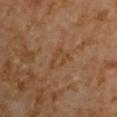Imaged during a routine full-body skin examination; the lesion was not biopsied and no histopathology is available. The tile uses cross-polarized illumination. On the right upper arm. The total-body-photography lesion software estimated an area of roughly 4 mm², an eccentricity of roughly 0.8, and a symmetry-axis asymmetry near 0.5. The software also gave a color-variation rating of about 0/10 and a peripheral color-asymmetry measure near 0. And it measured lesion-presence confidence of about 100/100. A male subject, aged around 60. Measured at roughly 3.5 mm in maximum diameter. A close-up tile cropped from a whole-body skin photograph, about 15 mm across.